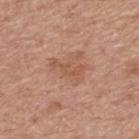workup = imaged on a skin check; not biopsied
image source = ~15 mm tile from a whole-body skin photo
illumination = white-light illumination
diameter = ~4 mm (longest diameter)
automated metrics = a mean CIELAB color near L≈54 a*≈22 b*≈32, about 7 CIELAB-L* units darker than the surrounding skin, and a normalized lesion–skin contrast near 6; internal color variation of about 1 on a 0–10 scale
body site = the upper back
patient = male, aged around 80